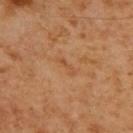No biopsy was performed on this lesion — it was imaged during a full skin examination and was not determined to be concerning. From the upper back. The patient is a male aged approximately 60. About 3 mm across. This is a cross-polarized tile. A close-up tile cropped from a whole-body skin photograph, about 15 mm across.